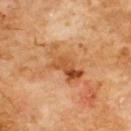{
  "biopsy_status": "not biopsied; imaged during a skin examination",
  "site": "chest",
  "automated_metrics": {
    "cielab_L": 52,
    "cielab_a": 25,
    "cielab_b": 40,
    "vs_skin_darker_L": 10.0,
    "vs_skin_contrast_norm": 7.5,
    "nevus_likeness_0_100": 0,
    "lesion_detection_confidence_0_100": 100
  },
  "patient": {
    "sex": "male",
    "age_approx": 60
  },
  "image": {
    "source": "total-body photography crop",
    "field_of_view_mm": 15
  },
  "lighting": "cross-polarized"
}